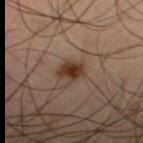notes: catalogued during a skin exam; not biopsied